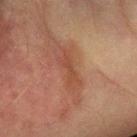follow-up: total-body-photography surveillance lesion; no biopsy
image-analysis metrics: an area of roughly 4 mm², a shape eccentricity near 0.95, and a shape-asymmetry score of about 0.45 (0 = symmetric); a lesion color around L≈38 a*≈21 b*≈27 in CIELAB, roughly 6 lightness units darker than nearby skin, and a normalized border contrast of about 5.5; a border-irregularity index near 5.5/10; a classifier nevus-likeness of about 0/100 and a lesion-detection confidence of about 60/100
subject: male, approximately 75 years of age
image: total-body-photography crop, ~15 mm field of view
lighting: cross-polarized illumination
site: the left forearm